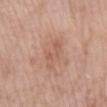Automated tile analysis of the lesion measured a lesion–skin lightness drop of about 7 and a lesion-to-skin contrast of about 4.5 (normalized; higher = more distinct). The software also gave a nevus-likeness score of about 0/100 and a detector confidence of about 100 out of 100 that the crop contains a lesion.
A female subject, approximately 70 years of age.
The lesion is on the left lower leg.
This image is a 15 mm lesion crop taken from a total-body photograph.
This is a white-light tile.